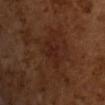Captured during whole-body skin photography for melanoma surveillance; the lesion was not biopsied. The recorded lesion diameter is about 3.5 mm. Automated image analysis of the tile measured a lesion color around L≈22 a*≈20 b*≈24 in CIELAB and roughly 4 lightness units darker than nearby skin. The analysis additionally found a classifier nevus-likeness of about 0/100 and lesion-presence confidence of about 100/100. A close-up tile cropped from a whole-body skin photograph, about 15 mm across. A male patient in their mid-60s. This is a cross-polarized tile.